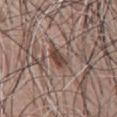notes=imaged on a skin check; not biopsied
subject=male, aged approximately 75
image source=total-body-photography crop, ~15 mm field of view
body site=the chest